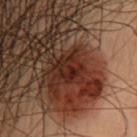Clinical impression: This lesion was catalogued during total-body skin photography and was not selected for biopsy. Clinical summary: Automated tile analysis of the lesion measured an area of roughly 7.5 mm², an outline eccentricity of about 0.4 (0 = round, 1 = elongated), and a symmetry-axis asymmetry near 0.2. It also reported a lesion-to-skin contrast of about 7.5 (normalized; higher = more distinct). It also reported a classifier nevus-likeness of about 0/100. A female subject, aged around 55. From the head or neck. This is a cross-polarized tile. Longest diameter approximately 3 mm. A roughly 15 mm field-of-view crop from a total-body skin photograph.